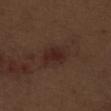Captured during whole-body skin photography for melanoma surveillance; the lesion was not biopsied. A male patient, aged 68 to 72. The tile uses white-light illumination. The lesion is located on the right thigh. A 15 mm close-up extracted from a 3D total-body photography capture.